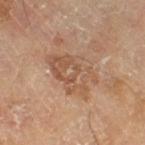notes — catalogued during a skin exam; not biopsied
body site — the right thigh
image source — ~15 mm tile from a whole-body skin photo
subject — male, in their mid- to late 60s